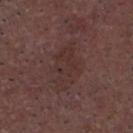Q: Was a biopsy performed?
A: total-body-photography surveillance lesion; no biopsy
Q: How was this image acquired?
A: 15 mm crop, total-body photography
Q: Where on the body is the lesion?
A: the head or neck
Q: Automated lesion metrics?
A: a shape eccentricity near 0.7; a lesion color around L≈31 a*≈18 b*≈19 in CIELAB, about 4 CIELAB-L* units darker than the surrounding skin, and a normalized lesion–skin contrast near 4.5; a classifier nevus-likeness of about 0/100 and lesion-presence confidence of about 100/100
Q: What are the patient's age and sex?
A: male, in their 50s
Q: What lighting was used for the tile?
A: white-light
Q: What is the lesion's diameter?
A: ~4.5 mm (longest diameter)